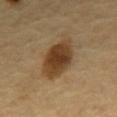| feature | finding |
|---|---|
| notes | total-body-photography surveillance lesion; no biopsy |
| lesion diameter | about 6 mm |
| illumination | cross-polarized |
| site | the mid back |
| patient | male, in their mid-60s |
| acquisition | ~15 mm crop, total-body skin-cancer survey |